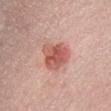workup: total-body-photography surveillance lesion; no biopsy
image source: ~15 mm tile from a whole-body skin photo
lesion diameter: about 4 mm
subject: female, about 55 years old
image-analysis metrics: an eccentricity of roughly 0.5 and a shape-asymmetry score of about 0.3 (0 = symmetric); internal color variation of about 5 on a 0–10 scale and a peripheral color-asymmetry measure near 1.5
location: the chest